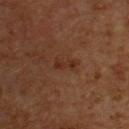Q: Is there a histopathology result?
A: catalogued during a skin exam; not biopsied
Q: What is the anatomic site?
A: the chest
Q: How large is the lesion?
A: ≈2.5 mm
Q: What did automated image analysis measure?
A: an outline eccentricity of about 0.95 (0 = round, 1 = elongated) and a symmetry-axis asymmetry near 0.4; a lesion color around L≈27 a*≈20 b*≈27 in CIELAB, roughly 6 lightness units darker than nearby skin, and a lesion-to-skin contrast of about 6.5 (normalized; higher = more distinct); a border-irregularity index near 4.5/10 and a color-variation rating of about 0/10; a nevus-likeness score of about 25/100 and a detector confidence of about 100 out of 100 that the crop contains a lesion
Q: Patient demographics?
A: male, aged approximately 55
Q: How was this image acquired?
A: ~15 mm crop, total-body skin-cancer survey